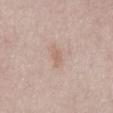The lesion was photographed on a routine skin check and not biopsied; there is no pathology result. The lesion-visualizer software estimated an area of roughly 3.5 mm², a shape eccentricity near 0.85, and a shape-asymmetry score of about 0.35 (0 = symmetric). The recorded lesion diameter is about 3 mm. The lesion is on the abdomen. A female patient aged around 50. Captured under white-light illumination. A lesion tile, about 15 mm wide, cut from a 3D total-body photograph.